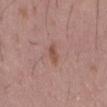The lesion was photographed on a routine skin check and not biopsied; there is no pathology result.
The lesion is located on the mid back.
The lesion's longest dimension is about 3 mm.
A male subject aged around 55.
A lesion tile, about 15 mm wide, cut from a 3D total-body photograph.
Captured under white-light illumination.